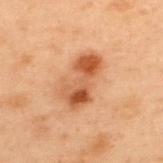Q: Was this lesion biopsied?
A: imaged on a skin check; not biopsied
Q: What is the imaging modality?
A: ~15 mm crop, total-body skin-cancer survey
Q: Who is the patient?
A: male, roughly 55 years of age
Q: What is the anatomic site?
A: the upper back
Q: Illumination type?
A: cross-polarized